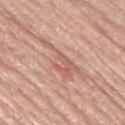Part of a total-body skin-imaging series; this lesion was reviewed on a skin check and was not flagged for biopsy. A region of skin cropped from a whole-body photographic capture, roughly 15 mm wide. On the left thigh. The subject is a male roughly 70 years of age.